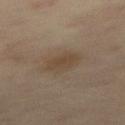Clinical impression:
Part of a total-body skin-imaging series; this lesion was reviewed on a skin check and was not flagged for biopsy.
Context:
Measured at roughly 3.5 mm in maximum diameter. A roughly 15 mm field-of-view crop from a total-body skin photograph. Imaged with cross-polarized lighting. A male patient, approximately 60 years of age. The lesion-visualizer software estimated a footprint of about 6.5 mm² and an outline eccentricity of about 0.75 (0 = round, 1 = elongated). The analysis additionally found an average lesion color of about L≈40 a*≈12 b*≈25 (CIELAB), about 6 CIELAB-L* units darker than the surrounding skin, and a normalized lesion–skin contrast near 6. The software also gave border irregularity of about 2 on a 0–10 scale, a within-lesion color-variation index near 1.5/10, and a peripheral color-asymmetry measure near 0.5. Located on the right thigh.